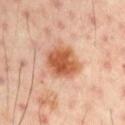{"lighting": "cross-polarized", "site": "left upper arm", "patient": {"sex": "male", "age_approx": 50}, "automated_metrics": {"cielab_L": 57, "cielab_a": 27, "cielab_b": 36, "vs_skin_darker_L": 14.0, "vs_skin_contrast_norm": 10.0, "border_irregularity_0_10": 2.0, "color_variation_0_10": 4.5, "nevus_likeness_0_100": 100, "lesion_detection_confidence_0_100": 100}, "lesion_size": {"long_diameter_mm_approx": 4.0}, "image": {"source": "total-body photography crop", "field_of_view_mm": 15}}